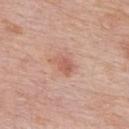biopsy status: total-body-photography surveillance lesion; no biopsy | lesion diameter: ≈3 mm | image source: ~15 mm crop, total-body skin-cancer survey | subject: male, about 75 years old | location: the upper back | illumination: white-light.